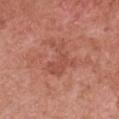acquisition — ~15 mm crop, total-body skin-cancer survey
site — the chest
subject — female, roughly 50 years of age
automated lesion analysis — a shape eccentricity near 0.75; an average lesion color of about L≈51 a*≈27 b*≈29 (CIELAB) and about 7 CIELAB-L* units darker than the surrounding skin
illumination — white-light illumination